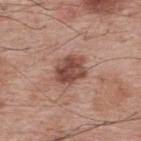Assessment: The lesion was tiled from a total-body skin photograph and was not biopsied. Context: This is a white-light tile. The subject is a male aged around 70. Longest diameter approximately 4 mm. The lesion is on the upper back. A 15 mm close-up extracted from a 3D total-body photography capture.